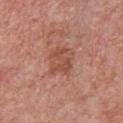No biopsy was performed on this lesion — it was imaged during a full skin examination and was not determined to be concerning. On the chest. A male patient roughly 70 years of age. Approximately 4 mm at its widest. The total-body-photography lesion software estimated roughly 8 lightness units darker than nearby skin and a normalized border contrast of about 6. And it measured a border-irregularity index near 3/10 and radial color variation of about 1.5. The software also gave a classifier nevus-likeness of about 0/100 and a detector confidence of about 100 out of 100 that the crop contains a lesion. This is a white-light tile. A close-up tile cropped from a whole-body skin photograph, about 15 mm across.